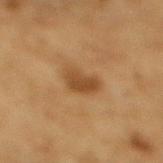Part of a total-body skin-imaging series; this lesion was reviewed on a skin check and was not flagged for biopsy.
Cropped from a total-body skin-imaging series; the visible field is about 15 mm.
The patient is a male aged around 85 or older.
An algorithmic analysis of the crop reported an area of roughly 6.5 mm², an eccentricity of roughly 0.8, and two-axis asymmetry of about 0.2. And it measured about 9 CIELAB-L* units darker than the surrounding skin. And it measured a border-irregularity rating of about 2/10, internal color variation of about 2.5 on a 0–10 scale, and a peripheral color-asymmetry measure near 1. It also reported a nevus-likeness score of about 50/100 and a lesion-detection confidence of about 100/100.
Captured under cross-polarized illumination.
The lesion is located on the mid back.